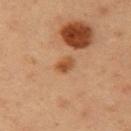{
  "biopsy_status": "not biopsied; imaged during a skin examination",
  "lesion_size": {
    "long_diameter_mm_approx": 2.5
  },
  "lighting": "cross-polarized",
  "site": "right upper arm",
  "patient": {
    "sex": "male",
    "age_approx": 35
  },
  "automated_metrics": {
    "area_mm2_approx": 3.5,
    "eccentricity": 0.7,
    "shape_asymmetry": 0.15,
    "cielab_L": 49,
    "cielab_a": 23,
    "cielab_b": 37,
    "vs_skin_darker_L": 10.0,
    "vs_skin_contrast_norm": 8.0,
    "border_irregularity_0_10": 1.5,
    "color_variation_0_10": 3.5,
    "peripheral_color_asymmetry": 1.5
  },
  "image": {
    "source": "total-body photography crop",
    "field_of_view_mm": 15
  }
}